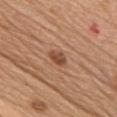A female patient, aged 58 to 62.
From the chest.
Measured at roughly 2.5 mm in maximum diameter.
A roughly 15 mm field-of-view crop from a total-body skin photograph.